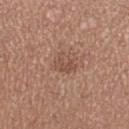Q: Is there a histopathology result?
A: catalogued during a skin exam; not biopsied
Q: What are the patient's age and sex?
A: female, aged approximately 30
Q: What is the anatomic site?
A: the leg
Q: What did automated image analysis measure?
A: an area of roughly 4 mm², an outline eccentricity of about 0.7 (0 = round, 1 = elongated), and a symmetry-axis asymmetry near 0.45; a nevus-likeness score of about 0/100 and a lesion-detection confidence of about 100/100
Q: What kind of image is this?
A: ~15 mm crop, total-body skin-cancer survey
Q: Illumination type?
A: white-light illumination
Q: Lesion size?
A: ≈3 mm